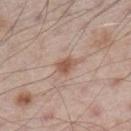patient: male, approximately 60 years of age
location: the left lower leg
imaging modality: total-body-photography crop, ~15 mm field of view
TBP lesion metrics: a lesion color around L≈55 a*≈19 b*≈28 in CIELAB and a normalized border contrast of about 8
tile lighting: white-light illumination